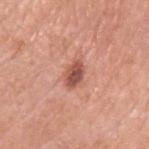Context: The lesion is on the left upper arm. The patient is a female aged approximately 70. A roughly 15 mm field-of-view crop from a total-body skin photograph.